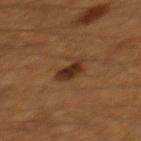Imaged during a routine full-body skin examination; the lesion was not biopsied and no histopathology is available.
Cropped from a total-body skin-imaging series; the visible field is about 15 mm.
The tile uses cross-polarized illumination.
A male patient, aged approximately 60.
The recorded lesion diameter is about 3.5 mm.
The lesion-visualizer software estimated a footprint of about 6 mm² and two-axis asymmetry of about 0.35. It also reported a within-lesion color-variation index near 3.5/10 and radial color variation of about 1. The software also gave a nevus-likeness score of about 95/100 and lesion-presence confidence of about 100/100.
Located on the mid back.